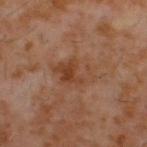Context: Imaged with cross-polarized lighting. From the back. This image is a 15 mm lesion crop taken from a total-body photograph. A male patient aged around 60. Measured at roughly 5 mm in maximum diameter. Automated tile analysis of the lesion measured a mean CIELAB color near L≈40 a*≈20 b*≈29, roughly 6 lightness units darker than nearby skin, and a normalized border contrast of about 6. And it measured a classifier nevus-likeness of about 0/100.